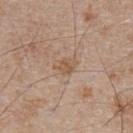Part of a total-body skin-imaging series; this lesion was reviewed on a skin check and was not flagged for biopsy.
A male subject, aged around 65.
This is a white-light tile.
Located on the chest.
A close-up tile cropped from a whole-body skin photograph, about 15 mm across.
An algorithmic analysis of the crop reported border irregularity of about 3 on a 0–10 scale, a within-lesion color-variation index near 2/10, and peripheral color asymmetry of about 0.5.